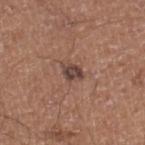Q: Was this lesion biopsied?
A: total-body-photography surveillance lesion; no biopsy
Q: How was this image acquired?
A: 15 mm crop, total-body photography
Q: Illumination type?
A: white-light illumination
Q: What is the anatomic site?
A: the right lower leg
Q: What are the patient's age and sex?
A: male, approximately 50 years of age